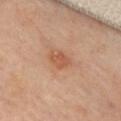Q: Was a biopsy performed?
A: catalogued during a skin exam; not biopsied
Q: What is the imaging modality?
A: ~15 mm crop, total-body skin-cancer survey
Q: How large is the lesion?
A: about 2.5 mm
Q: Where on the body is the lesion?
A: the front of the torso
Q: What did automated image analysis measure?
A: a footprint of about 4 mm², a shape eccentricity near 0.7, and two-axis asymmetry of about 0.3
Q: Patient demographics?
A: female, roughly 60 years of age